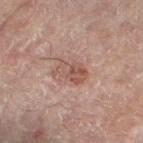acquisition: ~15 mm crop, total-body skin-cancer survey
subject: female, aged 78–82
anatomic site: the right leg